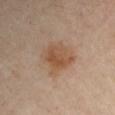{"biopsy_status": "not biopsied; imaged during a skin examination", "image": {"source": "total-body photography crop", "field_of_view_mm": 15}, "automated_metrics": {"cielab_L": 50, "cielab_a": 18, "cielab_b": 31, "vs_skin_darker_L": 8.0, "lesion_detection_confidence_0_100": 100}, "site": "left upper arm", "lesion_size": {"long_diameter_mm_approx": 4.0}, "patient": {"age_approx": 55}, "lighting": "cross-polarized"}